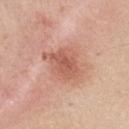biopsy status = total-body-photography surveillance lesion; no biopsy | automated lesion analysis = roughly 10 lightness units darker than nearby skin and a normalized lesion–skin contrast near 6.5; a nevus-likeness score of about 15/100 and a detector confidence of about 100 out of 100 that the crop contains a lesion | anatomic site = the chest | subject = female, aged 43–47 | acquisition = total-body-photography crop, ~15 mm field of view | illumination = white-light.